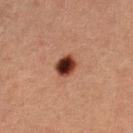Notes:
– follow-up — catalogued during a skin exam; not biopsied
– tile lighting — cross-polarized
– subject — female, aged 38–42
– automated lesion analysis — a lesion color around L≈30 a*≈21 b*≈25 in CIELAB; an automated nevus-likeness rating near 100 out of 100 and lesion-presence confidence of about 100/100
– site — the left lower leg
– size — ~2.5 mm (longest diameter)
– image — ~15 mm tile from a whole-body skin photo